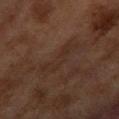• lesion diameter · ~5.5 mm (longest diameter)
• subject · female, in their 60s
• automated lesion analysis · an area of roughly 9 mm², an eccentricity of roughly 0.95, and two-axis asymmetry of about 0.5; a border-irregularity index near 6.5/10, a color-variation rating of about 2/10, and a peripheral color-asymmetry measure near 0.5; a classifier nevus-likeness of about 0/100 and lesion-presence confidence of about 60/100
• image · ~15 mm crop, total-body skin-cancer survey
• lighting · cross-polarized
• body site · the arm